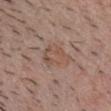{
  "site": "chest",
  "lighting": "white-light",
  "image": {
    "source": "total-body photography crop",
    "field_of_view_mm": 15
  },
  "lesion_size": {
    "long_diameter_mm_approx": 3.5
  },
  "patient": {
    "sex": "male",
    "age_approx": 40
  }
}